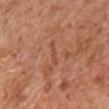Case summary:
– follow-up · no biopsy performed (imaged during a skin exam)
– automated lesion analysis · an area of roughly 2.5 mm² and an outline eccentricity of about 0.95 (0 = round, 1 = elongated); roughly 6 lightness units darker than nearby skin and a lesion-to-skin contrast of about 4.5 (normalized; higher = more distinct); a border-irregularity index near 6.5/10 and a peripheral color-asymmetry measure near 0
– anatomic site · the front of the torso
– tile lighting · white-light
– acquisition · ~15 mm tile from a whole-body skin photo
– subject · male, about 65 years old